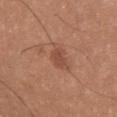Clinical impression: The lesion was tiled from a total-body skin photograph and was not biopsied. Context: Automated image analysis of the tile measured a footprint of about 3.5 mm² and a symmetry-axis asymmetry near 0.2. It also reported an average lesion color of about L≈48 a*≈25 b*≈30 (CIELAB). The software also gave an automated nevus-likeness rating near 40 out of 100 and a detector confidence of about 100 out of 100 that the crop contains a lesion. Cropped from a whole-body photographic skin survey; the tile spans about 15 mm. About 2.5 mm across. Imaged with white-light lighting. A male subject, about 25 years old. From the chest.